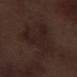The lesion was tiled from a total-body skin photograph and was not biopsied. Longest diameter approximately 7.5 mm. Cropped from a whole-body photographic skin survey; the tile spans about 15 mm. A male patient, aged approximately 70. The total-body-photography lesion software estimated roughly 5 lightness units darker than nearby skin and a normalized lesion–skin contrast near 6.5. The software also gave a classifier nevus-likeness of about 0/100 and a detector confidence of about 100 out of 100 that the crop contains a lesion. The lesion is located on the right lower leg.